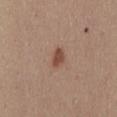Clinical impression:
The lesion was tiled from a total-body skin photograph and was not biopsied.
Acquisition and patient details:
This image is a 15 mm lesion crop taken from a total-body photograph. The lesion is on the mid back. The subject is a female in their 30s.